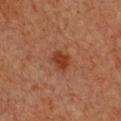Assessment:
Recorded during total-body skin imaging; not selected for excision or biopsy.
Image and clinical context:
On the front of the torso. A lesion tile, about 15 mm wide, cut from a 3D total-body photograph. Imaged with cross-polarized lighting. About 2.5 mm across. A female patient, in their 50s.